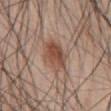follow-up: total-body-photography surveillance lesion; no biopsy | tile lighting: white-light illumination | patient: male, aged approximately 65 | automated metrics: an average lesion color of about L≈49 a*≈20 b*≈28 (CIELAB), a lesion–skin lightness drop of about 11, and a normalized lesion–skin contrast near 8.5; a classifier nevus-likeness of about 100/100 and lesion-presence confidence of about 100/100 | size: ~4 mm (longest diameter) | image: ~15 mm tile from a whole-body skin photo | location: the abdomen.